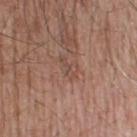Part of a total-body skin-imaging series; this lesion was reviewed on a skin check and was not flagged for biopsy. A male patient aged 53 to 57. The tile uses white-light illumination. The lesion is on the back. A region of skin cropped from a whole-body photographic capture, roughly 15 mm wide.